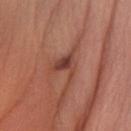<lesion>
  <biopsy_status>not biopsied; imaged during a skin examination</biopsy_status>
  <lighting>white-light</lighting>
  <site>left forearm</site>
  <lesion_size>
    <long_diameter_mm_approx>3.0</long_diameter_mm_approx>
  </lesion_size>
  <image>
    <source>total-body photography crop</source>
    <field_of_view_mm>15</field_of_view_mm>
  </image>
  <patient>
    <sex>female</sex>
    <age_approx>25</age_approx>
  </patient>
</lesion>